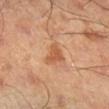{
  "biopsy_status": "not biopsied; imaged during a skin examination",
  "site": "right lower leg",
  "image": {
    "source": "total-body photography crop",
    "field_of_view_mm": 15
  },
  "lighting": "cross-polarized",
  "patient": {
    "sex": "male",
    "age_approx": 45
  },
  "automated_metrics": {
    "nevus_likeness_0_100": 0,
    "lesion_detection_confidence_0_100": 100
  },
  "lesion_size": {
    "long_diameter_mm_approx": 3.5
  }
}